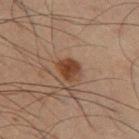workup: no biopsy performed (imaged during a skin exam).